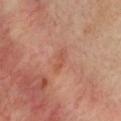{
  "biopsy_status": "not biopsied; imaged during a skin examination",
  "patient": {
    "sex": "female",
    "age_approx": 55
  },
  "site": "chest",
  "lighting": "cross-polarized",
  "lesion_size": {
    "long_diameter_mm_approx": 3.0
  },
  "automated_metrics": {
    "border_irregularity_0_10": 3.5,
    "color_variation_0_10": 0.0,
    "peripheral_color_asymmetry": 0.0
  },
  "image": {
    "source": "total-body photography crop",
    "field_of_view_mm": 15
  }
}